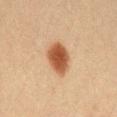Acquisition and patient details: Captured under cross-polarized illumination. A 15 mm close-up tile from a total-body photography series done for melanoma screening. The lesion is located on the lower back. A female patient aged 18 to 22.